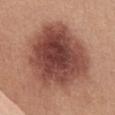No biopsy was performed on this lesion — it was imaged during a full skin examination and was not determined to be concerning. Located on the chest. Captured under white-light illumination. A 15 mm close-up extracted from a 3D total-body photography capture. A female patient, about 45 years old.